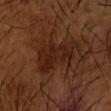Q: Was a biopsy performed?
A: no biopsy performed (imaged during a skin exam)
Q: Illumination type?
A: cross-polarized
Q: Automated lesion metrics?
A: a shape eccentricity near 0.8 and a shape-asymmetry score of about 0.4 (0 = symmetric)
Q: Lesion size?
A: ≈6 mm
Q: Lesion location?
A: the right forearm
Q: Who is the patient?
A: male, about 65 years old
Q: What is the imaging modality?
A: ~15 mm tile from a whole-body skin photo